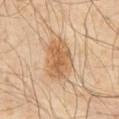The lesion was tiled from a total-body skin photograph and was not biopsied. A 15 mm crop from a total-body photograph taken for skin-cancer surveillance. Automated tile analysis of the lesion measured a lesion area of about 13 mm², an outline eccentricity of about 0.75 (0 = round, 1 = elongated), and a symmetry-axis asymmetry near 0.25. The software also gave a normalized border contrast of about 7.5. The software also gave an automated nevus-likeness rating near 80 out of 100 and lesion-presence confidence of about 100/100. A male subject aged 53–57. Measured at roughly 5 mm in maximum diameter. The lesion is on the abdomen. This is a cross-polarized tile.